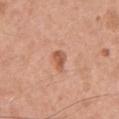biopsy status = total-body-photography surveillance lesion; no biopsy
subject = male, roughly 55 years of age
imaging modality = 15 mm crop, total-body photography
lesion diameter = ~2.5 mm (longest diameter)
location = the chest
lighting = white-light illumination